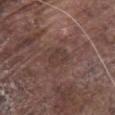notes=no biopsy performed (imaged during a skin exam); subject=male, roughly 80 years of age; tile lighting=white-light illumination; acquisition=15 mm crop, total-body photography; body site=the chest.